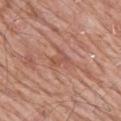This lesion was catalogued during total-body skin photography and was not selected for biopsy. Captured under white-light illumination. Cropped from a total-body skin-imaging series; the visible field is about 15 mm. The subject is a male aged 78–82. On the mid back. The lesion's longest dimension is about 2.5 mm.